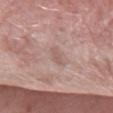Q: What lighting was used for the tile?
A: white-light illumination
Q: Where on the body is the lesion?
A: the arm
Q: What is the imaging modality?
A: ~15 mm crop, total-body skin-cancer survey
Q: How large is the lesion?
A: ≈2.5 mm
Q: Who is the patient?
A: female, in their 60s